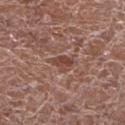Clinical impression: Recorded during total-body skin imaging; not selected for excision or biopsy. Background: The lesion is located on the left lower leg. A female subject aged 78–82. A 15 mm crop from a total-body photograph taken for skin-cancer surveillance. About 2.5 mm across. Imaged with white-light lighting.